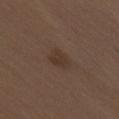This lesion was catalogued during total-body skin photography and was not selected for biopsy.
A 15 mm crop from a total-body photograph taken for skin-cancer surveillance.
The patient is a male aged approximately 70.
Captured under white-light illumination.
The lesion is on the right thigh.